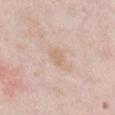{"biopsy_status": "not biopsied; imaged during a skin examination", "patient": {"sex": "female", "age_approx": 65}, "site": "chest", "image": {"source": "total-body photography crop", "field_of_view_mm": 15}}